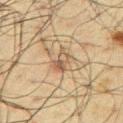Impression:
Captured during whole-body skin photography for melanoma surveillance; the lesion was not biopsied.
Acquisition and patient details:
The subject is a male roughly 60 years of age. A close-up tile cropped from a whole-body skin photograph, about 15 mm across. Captured under cross-polarized illumination. Approximately 3 mm at its widest. On the front of the torso.